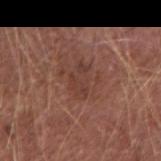* workup: no biopsy performed (imaged during a skin exam)
* location: the arm
* acquisition: ~15 mm tile from a whole-body skin photo
* subject: male, roughly 75 years of age
* tile lighting: white-light
* diameter: ~3.5 mm (longest diameter)
* TBP lesion metrics: a lesion area of about 5.5 mm², an eccentricity of roughly 0.8, and a symmetry-axis asymmetry near 0.35; roughly 6 lightness units darker than nearby skin and a normalized lesion–skin contrast near 5.5; a border-irregularity index near 4/10, a within-lesion color-variation index near 2/10, and radial color variation of about 0.5